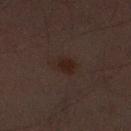Imaged during a routine full-body skin examination; the lesion was not biopsied and no histopathology is available. Approximately 3 mm at its widest. This is a cross-polarized tile. The patient is a male roughly 30 years of age. Automated tile analysis of the lesion measured a footprint of about 6 mm² and a shape-asymmetry score of about 0.25 (0 = symmetric). And it measured a border-irregularity rating of about 2/10, a within-lesion color-variation index near 2.5/10, and a peripheral color-asymmetry measure near 1. The analysis additionally found a classifier nevus-likeness of about 5/100 and a detector confidence of about 100 out of 100 that the crop contains a lesion. This image is a 15 mm lesion crop taken from a total-body photograph. The lesion is on the leg.